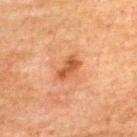<lesion>
  <biopsy_status>not biopsied; imaged during a skin examination</biopsy_status>
  <image>
    <source>total-body photography crop</source>
    <field_of_view_mm>15</field_of_view_mm>
  </image>
  <patient>
    <sex>male</sex>
    <age_approx>75</age_approx>
  </patient>
  <site>upper back</site>
  <lesion_size>
    <long_diameter_mm_approx>3.0</long_diameter_mm_approx>
  </lesion_size>
</lesion>